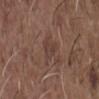{
  "biopsy_status": "not biopsied; imaged during a skin examination",
  "lighting": "white-light",
  "image": {
    "source": "total-body photography crop",
    "field_of_view_mm": 15
  },
  "patient": {
    "sex": "male",
    "age_approx": 50
  },
  "lesion_size": {
    "long_diameter_mm_approx": 2.5
  },
  "site": "chest"
}